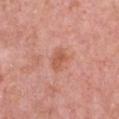workup: total-body-photography surveillance lesion; no biopsy | patient: female, approximately 40 years of age | imaging modality: 15 mm crop, total-body photography | body site: the chest.